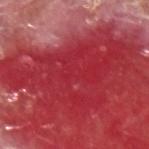Imaged during a routine full-body skin examination; the lesion was not biopsied and no histopathology is available. The lesion's longest dimension is about 16 mm. A male patient aged 63 to 67. Automated tile analysis of the lesion measured a lesion area of about 70 mm², a shape eccentricity near 0.85, and two-axis asymmetry of about 0.45. Imaged with white-light lighting. A region of skin cropped from a whole-body photographic capture, roughly 15 mm wide. The lesion is on the upper back.